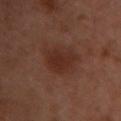Clinical impression:
Imaged during a routine full-body skin examination; the lesion was not biopsied and no histopathology is available.
Clinical summary:
Approximately 4.5 mm at its widest. A lesion tile, about 15 mm wide, cut from a 3D total-body photograph. Imaged with cross-polarized lighting. The lesion-visualizer software estimated an area of roughly 9.5 mm² and two-axis asymmetry of about 0.25. The analysis additionally found roughly 5 lightness units darker than nearby skin and a normalized lesion–skin contrast near 6.5. The analysis additionally found a border-irregularity rating of about 2.5/10, a within-lesion color-variation index near 2/10, and peripheral color asymmetry of about 1. It also reported an automated nevus-likeness rating near 60 out of 100. A female subject, about 55 years old. From the chest.